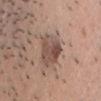Impression: Recorded during total-body skin imaging; not selected for excision or biopsy. Acquisition and patient details: The tile uses white-light illumination. From the head or neck. A male subject about 40 years old. Cropped from a whole-body photographic skin survey; the tile spans about 15 mm. The recorded lesion diameter is about 3.5 mm.